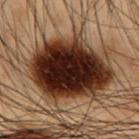Part of a total-body skin-imaging series; this lesion was reviewed on a skin check and was not flagged for biopsy. The patient is a male in their mid-50s. The tile uses cross-polarized illumination. A close-up tile cropped from a whole-body skin photograph, about 15 mm across. The recorded lesion diameter is about 9 mm. Located on the abdomen. Automated image analysis of the tile measured an area of roughly 47 mm², an eccentricity of roughly 0.65, and a symmetry-axis asymmetry near 0.15.